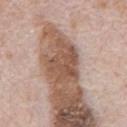notes: total-body-photography surveillance lesion; no biopsy | location: the chest | lesion diameter: ~5.5 mm (longest diameter) | subject: male, aged 68–72 | image source: 15 mm crop, total-body photography | image-analysis metrics: a footprint of about 14 mm² and a shape-asymmetry score of about 0.35 (0 = symmetric); border irregularity of about 4 on a 0–10 scale, a color-variation rating of about 4/10, and radial color variation of about 1.5; a classifier nevus-likeness of about 0/100.